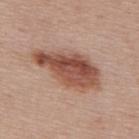Q: What is the imaging modality?
A: ~15 mm crop, total-body skin-cancer survey
Q: What is the anatomic site?
A: the upper back
Q: How large is the lesion?
A: about 8.5 mm
Q: Patient demographics?
A: female, in their 50s